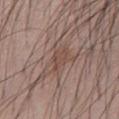{"biopsy_status": "not biopsied; imaged during a skin examination", "image": {"source": "total-body photography crop", "field_of_view_mm": 15}, "patient": {"sex": "male", "age_approx": 40}, "lighting": "white-light", "site": "chest"}